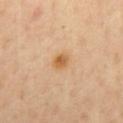- follow-up · no biopsy performed (imaged during a skin exam)
- image · ~15 mm crop, total-body skin-cancer survey
- subject · male, aged 53–57
- automated metrics · a lesion area of about 4 mm², an eccentricity of roughly 0.45, and two-axis asymmetry of about 0.15; about 9 CIELAB-L* units darker than the surrounding skin and a normalized lesion–skin contrast near 7.5; border irregularity of about 1 on a 0–10 scale, internal color variation of about 4.5 on a 0–10 scale, and peripheral color asymmetry of about 1.5; a classifier nevus-likeness of about 90/100 and a lesion-detection confidence of about 100/100
- site · the back
- illumination · cross-polarized illumination